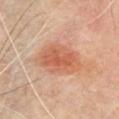Clinical impression: The lesion was photographed on a routine skin check and not biopsied; there is no pathology result. Acquisition and patient details: A male patient, aged 63 to 67. A 15 mm close-up tile from a total-body photography series done for melanoma screening. The tile uses cross-polarized illumination. Automated image analysis of the tile measured an area of roughly 17 mm² and a shape-asymmetry score of about 0.2 (0 = symmetric). It also reported an average lesion color of about L≈56 a*≈25 b*≈33 (CIELAB), about 9 CIELAB-L* units darker than the surrounding skin, and a lesion-to-skin contrast of about 7 (normalized; higher = more distinct). And it measured a classifier nevus-likeness of about 100/100 and lesion-presence confidence of about 100/100. The lesion is on the chest. Approximately 6 mm at its widest.